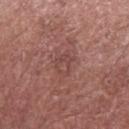Assessment:
Captured during whole-body skin photography for melanoma surveillance; the lesion was not biopsied.
Clinical summary:
This is a white-light tile. A 15 mm close-up extracted from a 3D total-body photography capture. About 2.5 mm across. The total-body-photography lesion software estimated an area of roughly 4.5 mm², an eccentricity of roughly 0.7, and a shape-asymmetry score of about 0.3 (0 = symmetric). And it measured an average lesion color of about L≈45 a*≈23 b*≈23 (CIELAB), roughly 6 lightness units darker than nearby skin, and a normalized lesion–skin contrast near 4.5. The analysis additionally found a detector confidence of about 95 out of 100 that the crop contains a lesion. A male patient, roughly 75 years of age. From the left forearm.